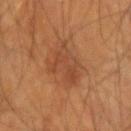{
  "biopsy_status": "not biopsied; imaged during a skin examination",
  "automated_metrics": {
    "area_mm2_approx": 11.0,
    "eccentricity": 0.7,
    "shape_asymmetry": 0.4,
    "vs_skin_darker_L": 6.0,
    "vs_skin_contrast_norm": 5.5,
    "nevus_likeness_0_100": 0,
    "lesion_detection_confidence_0_100": 100
  },
  "image": {
    "source": "total-body photography crop",
    "field_of_view_mm": 15
  },
  "patient": {
    "sex": "male",
    "age_approx": 65
  },
  "lesion_size": {
    "long_diameter_mm_approx": 4.5
  },
  "lighting": "cross-polarized",
  "site": "left forearm"
}